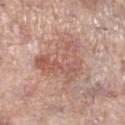The lesion was tiled from a total-body skin photograph and was not biopsied.
From the leg.
A close-up tile cropped from a whole-body skin photograph, about 15 mm across.
A female subject aged approximately 70.